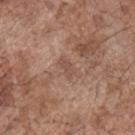Q: Automated lesion metrics?
A: a lesion color around L≈50 a*≈19 b*≈26 in CIELAB and roughly 7 lightness units darker than nearby skin; a classifier nevus-likeness of about 0/100 and lesion-presence confidence of about 95/100
Q: What are the patient's age and sex?
A: male, about 70 years old
Q: What is the anatomic site?
A: the chest
Q: How was the tile lit?
A: white-light
Q: What is the lesion's diameter?
A: ~2.5 mm (longest diameter)
Q: What is the imaging modality?
A: total-body-photography crop, ~15 mm field of view